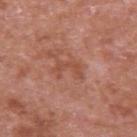workup: imaged on a skin check; not biopsied
automated metrics: a lesion area of about 4 mm², an eccentricity of roughly 0.85, and a symmetry-axis asymmetry near 0.6; a mean CIELAB color near L≈50 a*≈26 b*≈30, about 7 CIELAB-L* units darker than the surrounding skin, and a lesion-to-skin contrast of about 5.5 (normalized; higher = more distinct); border irregularity of about 8.5 on a 0–10 scale, internal color variation of about 0 on a 0–10 scale, and radial color variation of about 0
patient: male, aged 63–67
lesion size: ~3 mm (longest diameter)
illumination: white-light
image: ~15 mm crop, total-body skin-cancer survey
anatomic site: the arm